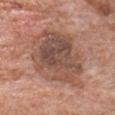  biopsy_status: not biopsied; imaged during a skin examination
  site: front of the torso
  image:
    source: total-body photography crop
    field_of_view_mm: 15
  patient:
    sex: male
    age_approx: 80
  lesion_size:
    long_diameter_mm_approx: 9.5
  automated_metrics:
    area_mm2_approx: 40.0
    eccentricity: 0.7
    shape_asymmetry: 0.15
    nevus_likeness_0_100: 5